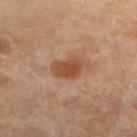This lesion was catalogued during total-body skin photography and was not selected for biopsy. Captured under cross-polarized illumination. From the left lower leg. A 15 mm crop from a total-body photograph taken for skin-cancer surveillance. The total-body-photography lesion software estimated a normalized border contrast of about 8. The lesion's longest dimension is about 3.5 mm. The patient is a female approximately 70 years of age.